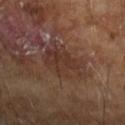Q: Is there a histopathology result?
A: total-body-photography surveillance lesion; no biopsy
Q: Patient demographics?
A: male, in their mid- to late 60s
Q: What is the anatomic site?
A: the left forearm
Q: How was this image acquired?
A: 15 mm crop, total-body photography